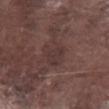follow-up = no biopsy performed (imaged during a skin exam)
illumination = white-light illumination
acquisition = 15 mm crop, total-body photography
lesion size = about 3.5 mm
location = the right lower leg
subject = male, in their mid-70s